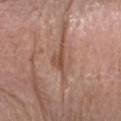Part of a total-body skin-imaging series; this lesion was reviewed on a skin check and was not flagged for biopsy.
The recorded lesion diameter is about 4.5 mm.
The tile uses white-light illumination.
A roughly 15 mm field-of-view crop from a total-body skin photograph.
From the head or neck.
The subject is a male in their mid- to late 70s.